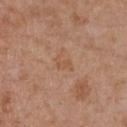| key | value |
|---|---|
| follow-up | no biopsy performed (imaged during a skin exam) |